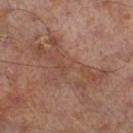Assessment:
Part of a total-body skin-imaging series; this lesion was reviewed on a skin check and was not flagged for biopsy.
Context:
A male subject, in their mid- to late 60s. A close-up tile cropped from a whole-body skin photograph, about 15 mm across. About 10 mm across. The lesion is on the right lower leg. The tile uses cross-polarized illumination. Automated tile analysis of the lesion measured lesion-presence confidence of about 60/100.